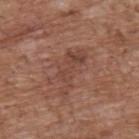biopsy status=total-body-photography surveillance lesion; no biopsy.